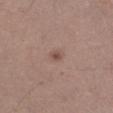Impression: Recorded during total-body skin imaging; not selected for excision or biopsy. Image and clinical context: A lesion tile, about 15 mm wide, cut from a 3D total-body photograph. About 1.5 mm across. The subject is a male aged around 45. This is a white-light tile. Located on the left lower leg.